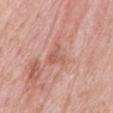<lesion>
<biopsy_status>not biopsied; imaged during a skin examination</biopsy_status>
<lesion_size>
  <long_diameter_mm_approx>3.5</long_diameter_mm_approx>
</lesion_size>
<image>
  <source>total-body photography crop</source>
  <field_of_view_mm>15</field_of_view_mm>
</image>
<lighting>white-light</lighting>
<site>mid back</site>
<patient>
  <sex>female</sex>
  <age_approx>60</age_approx>
</patient>
</lesion>